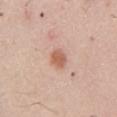Q: Was a biopsy performed?
A: no biopsy performed (imaged during a skin exam)
Q: What kind of image is this?
A: total-body-photography crop, ~15 mm field of view
Q: Patient demographics?
A: male, aged around 60
Q: Automated lesion metrics?
A: a footprint of about 4 mm², an outline eccentricity of about 0.75 (0 = round, 1 = elongated), and two-axis asymmetry of about 0.15
Q: What is the anatomic site?
A: the chest
Q: How large is the lesion?
A: ≈2.5 mm